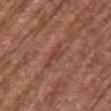Findings:
- size — ≈3 mm
- subject — female, aged 78–82
- acquisition — total-body-photography crop, ~15 mm field of view
- body site — the left upper arm